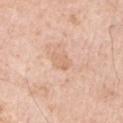The lesion was tiled from a total-body skin photograph and was not biopsied. The lesion-visualizer software estimated a footprint of about 4 mm² and a symmetry-axis asymmetry near 0.2. The analysis additionally found internal color variation of about 1 on a 0–10 scale and peripheral color asymmetry of about 0.5. The analysis additionally found a classifier nevus-likeness of about 0/100 and lesion-presence confidence of about 100/100. From the left upper arm. Imaged with white-light lighting. The recorded lesion diameter is about 2.5 mm. The subject is a male roughly 55 years of age. A 15 mm crop from a total-body photograph taken for skin-cancer surveillance.